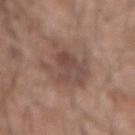| field | value |
|---|---|
| image source | 15 mm crop, total-body photography |
| patient | male, about 60 years old |
| anatomic site | the mid back |